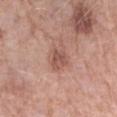Captured during whole-body skin photography for melanoma surveillance; the lesion was not biopsied.
A female patient, aged approximately 70.
The lesion is on the left forearm.
A 15 mm close-up extracted from a 3D total-body photography capture.
The tile uses white-light illumination.
Automated image analysis of the tile measured a lesion area of about 7 mm² and a symmetry-axis asymmetry near 0.15. The analysis additionally found a lesion color around L≈54 a*≈22 b*≈26 in CIELAB, a lesion–skin lightness drop of about 9, and a normalized lesion–skin contrast near 6.
Longest diameter approximately 3.5 mm.